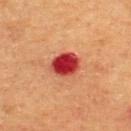Impression: Captured during whole-body skin photography for melanoma surveillance; the lesion was not biopsied. Context: Cropped from a whole-body photographic skin survey; the tile spans about 15 mm. From the upper back. Approximately 3.5 mm at its widest. The patient is a male approximately 60 years of age.